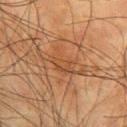Part of a total-body skin-imaging series; this lesion was reviewed on a skin check and was not flagged for biopsy. Measured at roughly 7.5 mm in maximum diameter. The lesion is located on the right upper arm. A male patient, in their mid- to late 70s. Imaged with cross-polarized lighting. A close-up tile cropped from a whole-body skin photograph, about 15 mm across.